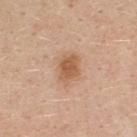The lesion was photographed on a routine skin check and not biopsied; there is no pathology result. The lesion is located on the upper back. A 15 mm crop from a total-body photograph taken for skin-cancer surveillance. The tile uses white-light illumination. The total-body-photography lesion software estimated an average lesion color of about L≈58 a*≈21 b*≈34 (CIELAB), roughly 11 lightness units darker than nearby skin, and a lesion-to-skin contrast of about 7.5 (normalized; higher = more distinct). It also reported a classifier nevus-likeness of about 85/100 and a detector confidence of about 100 out of 100 that the crop contains a lesion. A female patient roughly 40 years of age. Longest diameter approximately 3.5 mm.